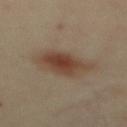Q: Was a biopsy performed?
A: catalogued during a skin exam; not biopsied
Q: Patient demographics?
A: female, in their 40s
Q: What is the anatomic site?
A: the mid back
Q: How large is the lesion?
A: about 5 mm
Q: Automated lesion metrics?
A: an automated nevus-likeness rating near 100 out of 100 and a detector confidence of about 100 out of 100 that the crop contains a lesion
Q: What is the imaging modality?
A: total-body-photography crop, ~15 mm field of view
Q: Illumination type?
A: cross-polarized